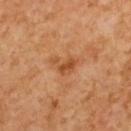<lesion>
  <biopsy_status>not biopsied; imaged during a skin examination</biopsy_status>
  <lesion_size>
    <long_diameter_mm_approx>3.5</long_diameter_mm_approx>
  </lesion_size>
  <patient>
    <sex>male</sex>
    <age_approx>60</age_approx>
  </patient>
  <automated_metrics>
    <vs_skin_darker_L>8.0</vs_skin_darker_L>
    <border_irregularity_0_10>5.5</border_irregularity_0_10>
    <color_variation_0_10>2.5</color_variation_0_10>
    <peripheral_color_asymmetry>1.0</peripheral_color_asymmetry>
    <nevus_likeness_0_100>0</nevus_likeness_0_100>
  </automated_metrics>
  <site>back</site>
  <image>
    <source>total-body photography crop</source>
    <field_of_view_mm>15</field_of_view_mm>
  </image>
  <lighting>cross-polarized</lighting>
</lesion>